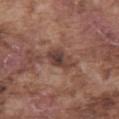Image and clinical context:
Approximately 3.5 mm at its widest. The patient is a male in their mid-70s. Located on the abdomen. A 15 mm crop from a total-body photograph taken for skin-cancer surveillance.